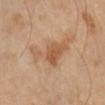| feature | finding |
|---|---|
| biopsy status | total-body-photography surveillance lesion; no biopsy |
| image source | ~15 mm crop, total-body skin-cancer survey |
| tile lighting | cross-polarized |
| lesion size | ~6.5 mm (longest diameter) |
| anatomic site | the right lower leg |
| patient | female, aged approximately 70 |